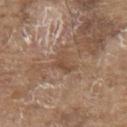Q: Was a biopsy performed?
A: no biopsy performed (imaged during a skin exam)
Q: Where on the body is the lesion?
A: the upper back
Q: Patient demographics?
A: male, aged 78 to 82
Q: What is the imaging modality?
A: ~15 mm tile from a whole-body skin photo
Q: What lighting was used for the tile?
A: white-light illumination
Q: What is the lesion's diameter?
A: ≈3 mm
Q: What did automated image analysis measure?
A: roughly 8 lightness units darker than nearby skin; a color-variation rating of about 2.5/10; a classifier nevus-likeness of about 0/100 and a detector confidence of about 60 out of 100 that the crop contains a lesion